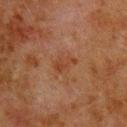workup — no biopsy performed (imaged during a skin exam) | patient — male, roughly 80 years of age | acquisition — 15 mm crop, total-body photography | site — the back.